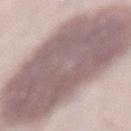Part of a total-body skin-imaging series; this lesion was reviewed on a skin check and was not flagged for biopsy. Located on the mid back. Automated tile analysis of the lesion measured an area of roughly 125 mm², a shape eccentricity near 0.8, and a symmetry-axis asymmetry near 0.15. The software also gave a mean CIELAB color near L≈59 a*≈15 b*≈17 and a lesion–skin lightness drop of about 16. It also reported a border-irregularity rating of about 2.5/10, a within-lesion color-variation index near 4.5/10, and a peripheral color-asymmetry measure near 1.5. And it measured a nevus-likeness score of about 5/100 and lesion-presence confidence of about 0/100. A lesion tile, about 15 mm wide, cut from a 3D total-body photograph. A male patient, aged around 55. The tile uses white-light illumination. The recorded lesion diameter is about 17 mm.